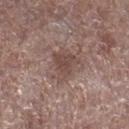Acquisition and patient details: The lesion's longest dimension is about 4 mm. The subject is a male in their 70s. Captured under white-light illumination. A roughly 15 mm field-of-view crop from a total-body skin photograph. The lesion is on the right lower leg.